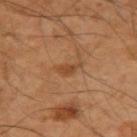Assessment: Recorded during total-body skin imaging; not selected for excision or biopsy. Background: A region of skin cropped from a whole-body photographic capture, roughly 15 mm wide. A male patient, in their 50s. The lesion is on the left arm. Longest diameter approximately 3 mm.